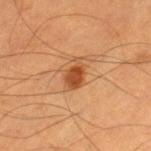| key | value |
|---|---|
| patient | male, aged 53 to 57 |
| image-analysis metrics | a footprint of about 5.5 mm² and a shape-asymmetry score of about 0.3 (0 = symmetric); an average lesion color of about L≈49 a*≈27 b*≈40 (CIELAB); a border-irregularity rating of about 3/10, a within-lesion color-variation index near 2.5/10, and radial color variation of about 1; a nevus-likeness score of about 95/100 and a detector confidence of about 100 out of 100 that the crop contains a lesion |
| imaging modality | 15 mm crop, total-body photography |
| lighting | cross-polarized |
| anatomic site | the left thigh |
| lesion size | ~3.5 mm (longest diameter) |